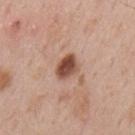Impression: Part of a total-body skin-imaging series; this lesion was reviewed on a skin check and was not flagged for biopsy. Context: A male subject, about 55 years old. Imaged with white-light lighting. A 15 mm close-up tile from a total-body photography series done for melanoma screening. On the mid back.